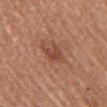biopsy status = imaged on a skin check; not biopsied.